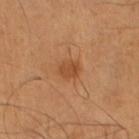biopsy_status: not biopsied; imaged during a skin examination
site: left lower leg
automated_metrics:
  area_mm2_approx: 4.5
  eccentricity: 0.5
  shape_asymmetry: 0.2
  vs_skin_darker_L: 8.0
lighting: cross-polarized
patient:
  sex: male
  age_approx: 55
lesion_size:
  long_diameter_mm_approx: 2.5
image:
  source: total-body photography crop
  field_of_view_mm: 15